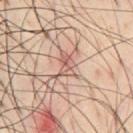{"biopsy_status": "not biopsied; imaged during a skin examination", "lighting": "cross-polarized", "lesion_size": {"long_diameter_mm_approx": 3.5}, "image": {"source": "total-body photography crop", "field_of_view_mm": 15}, "automated_metrics": {"cielab_L": 58, "cielab_a": 20, "cielab_b": 25, "vs_skin_darker_L": 10.0, "vs_skin_contrast_norm": 6.5, "border_irregularity_0_10": 5.5, "color_variation_0_10": 4.0, "peripheral_color_asymmetry": 1.5, "nevus_likeness_0_100": 0, "lesion_detection_confidence_0_100": 15}, "patient": {"sex": "male", "age_approx": 45}, "site": "chest"}